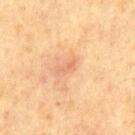Imaged during a routine full-body skin examination; the lesion was not biopsied and no histopathology is available. A 15 mm close-up tile from a total-body photography series done for melanoma screening. The lesion is on the back. The total-body-photography lesion software estimated a lesion area of about 2.5 mm², a shape eccentricity near 0.9, and a shape-asymmetry score of about 0.55 (0 = symmetric). It also reported an automated nevus-likeness rating near 0 out of 100 and a detector confidence of about 100 out of 100 that the crop contains a lesion. A male subject, aged approximately 70.